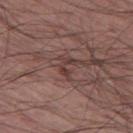No biopsy was performed on this lesion — it was imaged during a full skin examination and was not determined to be concerning.
The total-body-photography lesion software estimated a footprint of about 3.5 mm². And it measured a border-irregularity index near 8/10, a color-variation rating of about 0/10, and radial color variation of about 0.
Imaged with white-light lighting.
A male patient, aged around 60.
This image is a 15 mm lesion crop taken from a total-body photograph.
Approximately 3 mm at its widest.
From the leg.